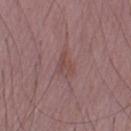Recorded during total-body skin imaging; not selected for excision or biopsy.
This image is a 15 mm lesion crop taken from a total-body photograph.
A male patient about 50 years old.
From the left upper arm.